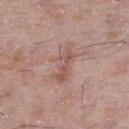Impression: Captured during whole-body skin photography for melanoma surveillance; the lesion was not biopsied. Background: The lesion is located on the right thigh. Measured at roughly 5 mm in maximum diameter. Imaged with white-light lighting. A male patient, roughly 50 years of age. A 15 mm close-up tile from a total-body photography series done for melanoma screening.